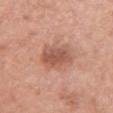Impression: Captured during whole-body skin photography for melanoma surveillance; the lesion was not biopsied. Context: This is a white-light tile. A lesion tile, about 15 mm wide, cut from a 3D total-body photograph. The recorded lesion diameter is about 4 mm. On the upper back. A female patient, aged 58 to 62.